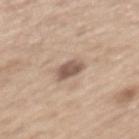notes=total-body-photography surveillance lesion; no biopsy
image source=15 mm crop, total-body photography
tile lighting=white-light
anatomic site=the back
size=≈3 mm
patient=male, aged approximately 70